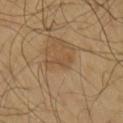Part of a total-body skin-imaging series; this lesion was reviewed on a skin check and was not flagged for biopsy. From the right lower leg. This image is a 15 mm lesion crop taken from a total-body photograph. Measured at roughly 3.5 mm in maximum diameter. Automated image analysis of the tile measured a lesion color around L≈49 a*≈17 b*≈34 in CIELAB. The software also gave a border-irregularity index near 6/10, a color-variation rating of about 0/10, and radial color variation of about 0. The software also gave an automated nevus-likeness rating near 85 out of 100 and a detector confidence of about 100 out of 100 that the crop contains a lesion. A male patient, in their mid-60s.